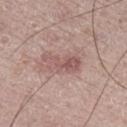Impression:
The lesion was photographed on a routine skin check and not biopsied; there is no pathology result.
Acquisition and patient details:
The tile uses white-light illumination. A male patient aged around 65. Longest diameter approximately 4.5 mm. From the right lower leg. Cropped from a whole-body photographic skin survey; the tile spans about 15 mm.